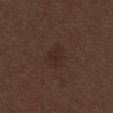Notes:
– workup — no biopsy performed (imaged during a skin exam)
– diameter — about 3 mm
– illumination — white-light illumination
– subject — male, roughly 70 years of age
– anatomic site — the abdomen
– image-analysis metrics — an area of roughly 7 mm², an outline eccentricity of about 0.55 (0 = round, 1 = elongated), and a shape-asymmetry score of about 0.15 (0 = symmetric); an average lesion color of about L≈26 a*≈14 b*≈21 (CIELAB) and about 4 CIELAB-L* units darker than the surrounding skin; an automated nevus-likeness rating near 10 out of 100 and a detector confidence of about 100 out of 100 that the crop contains a lesion
– acquisition — ~15 mm crop, total-body skin-cancer survey